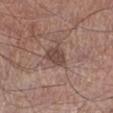The lesion was photographed on a routine skin check and not biopsied; there is no pathology result. The patient is a male aged 68–72. On the leg. The recorded lesion diameter is about 3 mm. Captured under white-light illumination. A close-up tile cropped from a whole-body skin photograph, about 15 mm across.